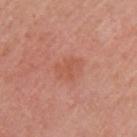Impression:
This lesion was catalogued during total-body skin photography and was not selected for biopsy.
Context:
The lesion's longest dimension is about 3 mm. The lesion is located on the arm. Cropped from a whole-body photographic skin survey; the tile spans about 15 mm. A female subject roughly 50 years of age.